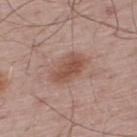Located on the upper back. A 15 mm close-up tile from a total-body photography series done for melanoma screening. About 5 mm across. A male subject approximately 65 years of age.